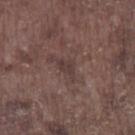biopsy_status: not biopsied; imaged during a skin examination
site: left lower leg
lighting: white-light
lesion_size:
  long_diameter_mm_approx: 2.5
patient:
  sex: male
  age_approx: 75
image:
  source: total-body photography crop
  field_of_view_mm: 15